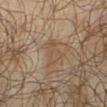A male subject roughly 65 years of age. This is a cross-polarized tile. Approximately 3.5 mm at its widest. The lesion is located on the left lower leg. A 15 mm close-up tile from a total-body photography series done for melanoma screening.